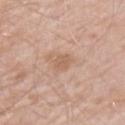biopsy_status: not biopsied; imaged during a skin examination
image:
  source: total-body photography crop
  field_of_view_mm: 15
automated_metrics:
  cielab_L: 60
  cielab_a: 19
  cielab_b: 31
  vs_skin_darker_L: 8.0
  nevus_likeness_0_100: 10
  lesion_detection_confidence_0_100: 100
site: right upper arm
lighting: white-light
patient:
  sex: male
  age_approx: 60